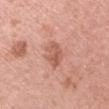• follow-up: catalogued during a skin exam; not biopsied
• lighting: white-light illumination
• image source: 15 mm crop, total-body photography
• automated lesion analysis: a mean CIELAB color near L≈58 a*≈25 b*≈31 and roughly 10 lightness units darker than nearby skin; a border-irregularity index near 2.5/10, internal color variation of about 4 on a 0–10 scale, and a peripheral color-asymmetry measure near 1.5
• lesion diameter: about 3.5 mm
• location: the left upper arm
• patient: female, in their mid-40s